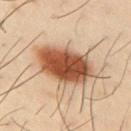follow-up: imaged on a skin check; not biopsied
location: the arm
tile lighting: cross-polarized
subject: male, approximately 40 years of age
imaging modality: total-body-photography crop, ~15 mm field of view
TBP lesion metrics: an area of roughly 26 mm² and a symmetry-axis asymmetry near 0.15; border irregularity of about 2.5 on a 0–10 scale and a within-lesion color-variation index near 7.5/10; an automated nevus-likeness rating near 100 out of 100 and a lesion-detection confidence of about 100/100
lesion diameter: about 8 mm